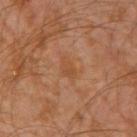| field | value |
|---|---|
| workup | imaged on a skin check; not biopsied |
| patient | male, aged around 30 |
| site | the left arm |
| size | about 3 mm |
| imaging modality | total-body-photography crop, ~15 mm field of view |